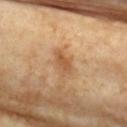workup=catalogued during a skin exam; not biopsied
patient=female, aged 73–77
site=the chest
lighting=cross-polarized illumination
imaging modality=~15 mm tile from a whole-body skin photo